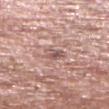notes = no biopsy performed (imaged during a skin exam)
lesion diameter = ≈3 mm
automated metrics = a mean CIELAB color near L≈57 a*≈19 b*≈22, about 8 CIELAB-L* units darker than the surrounding skin, and a normalized lesion–skin contrast near 6; border irregularity of about 4 on a 0–10 scale and a within-lesion color-variation index near 5.5/10; a nevus-likeness score of about 0/100
patient = male, aged approximately 55
tile lighting = white-light
imaging modality = ~15 mm crop, total-body skin-cancer survey
location = the right lower leg